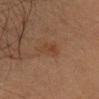No biopsy was performed on this lesion — it was imaged during a full skin examination and was not determined to be concerning. A 15 mm close-up extracted from a 3D total-body photography capture. Measured at roughly 3 mm in maximum diameter. A female subject aged around 45. From the head or neck. Imaged with cross-polarized lighting. Automated image analysis of the tile measured roughly 5 lightness units darker than nearby skin and a normalized lesion–skin contrast near 5.5. It also reported an automated nevus-likeness rating near 10 out of 100 and a lesion-detection confidence of about 100/100.